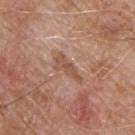automated metrics: a mean CIELAB color near L≈52 a*≈21 b*≈30, about 8 CIELAB-L* units darker than the surrounding skin, and a lesion-to-skin contrast of about 6 (normalized; higher = more distinct)
site: the upper back
patient: male, in their 80s
illumination: white-light illumination
image source: 15 mm crop, total-body photography